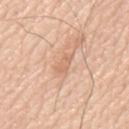Assessment: The lesion was photographed on a routine skin check and not biopsied; there is no pathology result. Clinical summary: The recorded lesion diameter is about 2.5 mm. From the mid back. Cropped from a whole-body photographic skin survey; the tile spans about 15 mm. This is a white-light tile. A male subject, in their 80s.